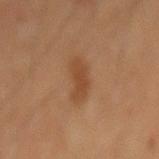Q: Was a biopsy performed?
A: no biopsy performed (imaged during a skin exam)
Q: Lesion location?
A: the abdomen
Q: What did automated image analysis measure?
A: a lesion color around L≈40 a*≈20 b*≈31 in CIELAB, a lesion–skin lightness drop of about 7, and a normalized lesion–skin contrast near 6.5; a border-irregularity rating of about 4.5/10, a within-lesion color-variation index near 2/10, and peripheral color asymmetry of about 0.5; a nevus-likeness score of about 50/100 and a lesion-detection confidence of about 100/100
Q: How was this image acquired?
A: total-body-photography crop, ~15 mm field of view
Q: Lesion size?
A: ≈3.5 mm
Q: What are the patient's age and sex?
A: male, aged around 60
Q: Illumination type?
A: cross-polarized